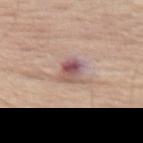Recorded during total-body skin imaging; not selected for excision or biopsy. Located on the abdomen. Longest diameter approximately 3 mm. A male subject in their 80s. Captured under white-light illumination. A lesion tile, about 15 mm wide, cut from a 3D total-body photograph.